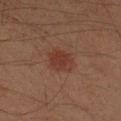The lesion was photographed on a routine skin check and not biopsied; there is no pathology result. A 15 mm close-up extracted from a 3D total-body photography capture. The lesion is on the left forearm. The subject is a male in their mid- to late 40s. Automated tile analysis of the lesion measured an average lesion color of about L≈29 a*≈19 b*≈22 (CIELAB) and about 6 CIELAB-L* units darker than the surrounding skin. The tile uses cross-polarized illumination.